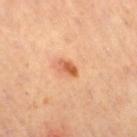* biopsy status · catalogued during a skin exam; not biopsied
* lighting · cross-polarized
* subject · female, in their 40s
* image · total-body-photography crop, ~15 mm field of view
* diameter · about 2.5 mm
* location · the right thigh